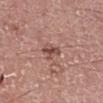| feature | finding |
|---|---|
| workup | total-body-photography surveillance lesion; no biopsy |
| lighting | white-light |
| location | the leg |
| acquisition | total-body-photography crop, ~15 mm field of view |
| size | ≈3 mm |
| patient | male, approximately 60 years of age |